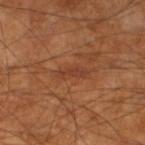Case summary:
* notes: total-body-photography surveillance lesion; no biopsy
* diameter: about 3.5 mm
* tile lighting: cross-polarized
* patient: male, in their 60s
* acquisition: ~15 mm crop, total-body skin-cancer survey
* site: the leg